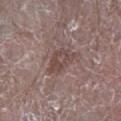biopsy status = total-body-photography surveillance lesion; no biopsy | acquisition = 15 mm crop, total-body photography | patient = male, roughly 65 years of age | body site = the right lower leg.